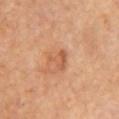| feature | finding |
|---|---|
| notes | no biopsy performed (imaged during a skin exam) |
| size | about 2.5 mm |
| anatomic site | the chest |
| patient | female, about 70 years old |
| imaging modality | total-body-photography crop, ~15 mm field of view |